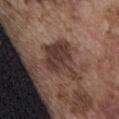The lesion was tiled from a total-body skin photograph and was not biopsied.
Cropped from a total-body skin-imaging series; the visible field is about 15 mm.
Longest diameter approximately 5.5 mm.
On the abdomen.
This is a white-light tile.
A male patient, about 75 years old.